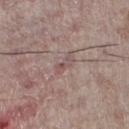Longest diameter approximately 3 mm. On the left lower leg. The total-body-photography lesion software estimated a lesion area of about 3 mm² and two-axis asymmetry of about 0.3. And it measured a border-irregularity rating of about 3/10, a within-lesion color-variation index near 0.5/10, and radial color variation of about 0. It also reported a classifier nevus-likeness of about 0/100 and a lesion-detection confidence of about 60/100. The patient is a male in their mid- to late 50s. A lesion tile, about 15 mm wide, cut from a 3D total-body photograph.